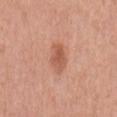Clinical impression:
The lesion was tiled from a total-body skin photograph and was not biopsied.
Background:
A 15 mm close-up tile from a total-body photography series done for melanoma screening. Captured under white-light illumination. From the mid back. A male subject roughly 70 years of age. The recorded lesion diameter is about 4 mm.